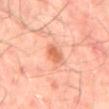Impression:
Part of a total-body skin-imaging series; this lesion was reviewed on a skin check and was not flagged for biopsy.
Clinical summary:
Imaged with cross-polarized lighting. The lesion is located on the mid back. A male patient approximately 50 years of age. Cropped from a total-body skin-imaging series; the visible field is about 15 mm.